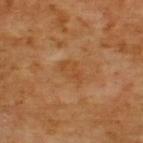Part of a total-body skin-imaging series; this lesion was reviewed on a skin check and was not flagged for biopsy.
Captured under cross-polarized illumination.
About 3 mm across.
A roughly 15 mm field-of-view crop from a total-body skin photograph.
The lesion is on the upper back.
A male subject, aged 63 to 67.
Automated image analysis of the tile measured a lesion area of about 4.5 mm² and a shape-asymmetry score of about 0.3 (0 = symmetric).